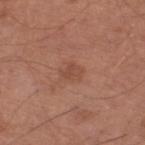<record>
<biopsy_status>not biopsied; imaged during a skin examination</biopsy_status>
<lighting>white-light</lighting>
<automated_metrics>
  <border_irregularity_0_10>3.5</border_irregularity_0_10>
  <color_variation_0_10>1.0</color_variation_0_10>
  <peripheral_color_asymmetry>0.5</peripheral_color_asymmetry>
  <nevus_likeness_0_100>20</nevus_likeness_0_100>
</automated_metrics>
<site>right thigh</site>
<patient>
  <sex>male</sex>
  <age_approx>65</age_approx>
</patient>
<image>
  <source>total-body photography crop</source>
  <field_of_view_mm>15</field_of_view_mm>
</image>
<lesion_size>
  <long_diameter_mm_approx>2.5</long_diameter_mm_approx>
</lesion_size>
</record>